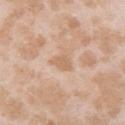  biopsy_status: not biopsied; imaged during a skin examination
  image:
    source: total-body photography crop
    field_of_view_mm: 15
  lesion_size:
    long_diameter_mm_approx: 2.5
  lighting: white-light
  site: arm
  patient:
    sex: female
    age_approx: 25